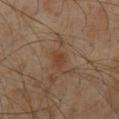{
  "biopsy_status": "not biopsied; imaged during a skin examination",
  "image": {
    "source": "total-body photography crop",
    "field_of_view_mm": 15
  },
  "lesion_size": {
    "long_diameter_mm_approx": 2.5
  },
  "patient": {
    "sex": "male",
    "age_approx": 60
  },
  "site": "abdomen"
}